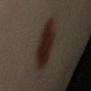biopsy status=catalogued during a skin exam; not biopsied | size=≈6.5 mm | tile lighting=cross-polarized illumination | body site=the left arm | subject=female, approximately 40 years of age | imaging modality=total-body-photography crop, ~15 mm field of view.